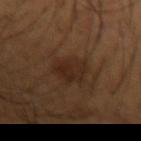Clinical impression:
Imaged during a routine full-body skin examination; the lesion was not biopsied and no histopathology is available.
Acquisition and patient details:
Cropped from a total-body skin-imaging series; the visible field is about 15 mm. The patient is a male aged around 65. Longest diameter approximately 3.5 mm. This is a cross-polarized tile. On the arm.